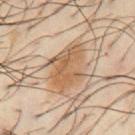Case summary:
- follow-up — total-body-photography surveillance lesion; no biopsy
- location — the chest
- patient — male, about 40 years old
- size — about 5.5 mm
- lighting — cross-polarized illumination
- image — 15 mm crop, total-body photography